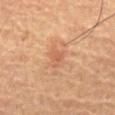  biopsy_status: not biopsied; imaged during a skin examination
  patient:
    sex: male
    age_approx: 55
  automated_metrics:
    area_mm2_approx: 1.0
    eccentricity: 0.7
    shape_asymmetry: 0.25
    cielab_L: 51
    cielab_a: 24
    cielab_b: 32
    vs_skin_darker_L: 6.0
    color_variation_0_10: 0.0
    peripheral_color_asymmetry: 0.0
    nevus_likeness_0_100: 0
    lesion_detection_confidence_0_100: 100
  image:
    source: total-body photography crop
    field_of_view_mm: 15
  site: chest
  lighting: cross-polarized